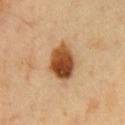Q: Was this lesion biopsied?
A: catalogued during a skin exam; not biopsied
Q: Who is the patient?
A: male, in their 50s
Q: Where on the body is the lesion?
A: the chest
Q: How was this image acquired?
A: ~15 mm crop, total-body skin-cancer survey
Q: Illumination type?
A: cross-polarized
Q: What is the lesion's diameter?
A: ≈4.5 mm